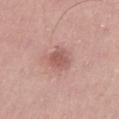Notes:
– follow-up: no biopsy performed (imaged during a skin exam)
– acquisition: total-body-photography crop, ~15 mm field of view
– automated lesion analysis: a footprint of about 6.5 mm² and an eccentricity of roughly 0.65; an average lesion color of about L≈56 a*≈24 b*≈24 (CIELAB), roughly 10 lightness units darker than nearby skin, and a lesion-to-skin contrast of about 6.5 (normalized; higher = more distinct); border irregularity of about 2 on a 0–10 scale, a color-variation rating of about 2/10, and radial color variation of about 0.5
– lesion diameter: ~3 mm (longest diameter)
– patient: female, aged 38–42
– illumination: white-light illumination
– site: the left thigh